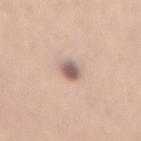Notes:
- follow-up: total-body-photography surveillance lesion; no biopsy